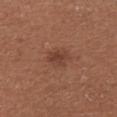Case summary:
– follow-up: no biopsy performed (imaged during a skin exam)
– TBP lesion metrics: a nevus-likeness score of about 45/100
– lighting: white-light illumination
– body site: the right forearm
– lesion size: about 3 mm
– image source: 15 mm crop, total-body photography
– subject: female, in their mid- to late 30s